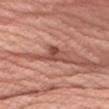Background: A close-up tile cropped from a whole-body skin photograph, about 15 mm across. A female subject, in their mid- to late 50s. About 2.5 mm across. This is a white-light tile. Located on the right upper arm.